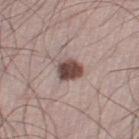{"biopsy_status": "not biopsied; imaged during a skin examination", "image": {"source": "total-body photography crop", "field_of_view_mm": 15}, "patient": {"sex": "male", "age_approx": 35}, "site": "left thigh", "lesion_size": {"long_diameter_mm_approx": 2.5}}